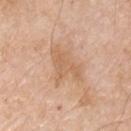Assessment:
Captured during whole-body skin photography for melanoma surveillance; the lesion was not biopsied.
Clinical summary:
An algorithmic analysis of the crop reported a lesion color around L≈63 a*≈20 b*≈34 in CIELAB, roughly 7 lightness units darker than nearby skin, and a normalized border contrast of about 5. A male patient, approximately 65 years of age. The recorded lesion diameter is about 4.5 mm. On the chest. A close-up tile cropped from a whole-body skin photograph, about 15 mm across. Captured under white-light illumination.